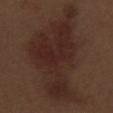Case summary:
• notes — catalogued during a skin exam; not biopsied
• site — the left thigh
• acquisition — ~15 mm crop, total-body skin-cancer survey
• lesion size — ≈13.5 mm
• subject — male, in their 70s
• lighting — white-light illumination